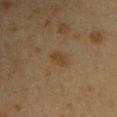follow-up — no biopsy performed (imaged during a skin exam) | diameter — ≈2.5 mm | site — the right upper arm | image source — total-body-photography crop, ~15 mm field of view | lighting — cross-polarized | automated lesion analysis — a lesion area of about 3.5 mm², an outline eccentricity of about 0.85 (0 = round, 1 = elongated), and a symmetry-axis asymmetry near 0.25; a border-irregularity rating of about 2/10 and a color-variation rating of about 1/10 | subject — female, aged 38 to 42.